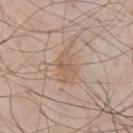{
  "biopsy_status": "not biopsied; imaged during a skin examination",
  "lesion_size": {
    "long_diameter_mm_approx": 5.0
  },
  "lighting": "white-light",
  "site": "chest",
  "image": {
    "source": "total-body photography crop",
    "field_of_view_mm": 15
  },
  "patient": {
    "sex": "male",
    "age_approx": 55
  }
}